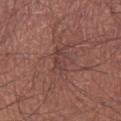Image and clinical context: Cropped from a whole-body photographic skin survey; the tile spans about 15 mm. From the right lower leg. The recorded lesion diameter is about 3 mm. The patient is a male aged 53–57.